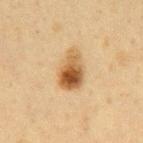The lesion was photographed on a routine skin check and not biopsied; there is no pathology result.
Automated image analysis of the tile measured an eccentricity of roughly 0.8 and two-axis asymmetry of about 0.25. The analysis additionally found a within-lesion color-variation index near 9.5/10 and radial color variation of about 3. The software also gave lesion-presence confidence of about 100/100.
The lesion is located on the mid back.
A 15 mm crop from a total-body photograph taken for skin-cancer surveillance.
The tile uses cross-polarized illumination.
Longest diameter approximately 4.5 mm.
The subject is a male about 60 years old.